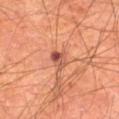– follow-up · no biopsy performed (imaged during a skin exam)
– subject · male, in their mid- to late 60s
– automated metrics · a footprint of about 3.5 mm², an eccentricity of roughly 0.75, and two-axis asymmetry of about 0.3; a border-irregularity index near 3/10 and peripheral color asymmetry of about 4; a nevus-likeness score of about 55/100 and a detector confidence of about 100 out of 100 that the crop contains a lesion
– size · about 2.5 mm
– body site · the leg
– imaging modality · ~15 mm tile from a whole-body skin photo